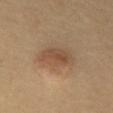| key | value |
|---|---|
| biopsy status | catalogued during a skin exam; not biopsied |
| diameter | ~4 mm (longest diameter) |
| location | the mid back |
| image source | 15 mm crop, total-body photography |
| subject | male, aged approximately 55 |
| tile lighting | cross-polarized |